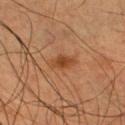Imaged during a routine full-body skin examination; the lesion was not biopsied and no histopathology is available. The lesion-visualizer software estimated a footprint of about 4 mm² and a shape-asymmetry score of about 0.25 (0 = symmetric). The analysis additionally found a border-irregularity index near 3/10, internal color variation of about 2.5 on a 0–10 scale, and radial color variation of about 0.5. It also reported a nevus-likeness score of about 90/100 and a lesion-detection confidence of about 100/100. Approximately 2.5 mm at its widest. A roughly 15 mm field-of-view crop from a total-body skin photograph. Located on the right lower leg. A male patient, in their mid- to late 40s. Captured under cross-polarized illumination.